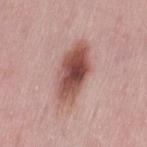Part of a total-body skin-imaging series; this lesion was reviewed on a skin check and was not flagged for biopsy.
A female patient aged approximately 50.
A 15 mm close-up tile from a total-body photography series done for melanoma screening.
This is a white-light tile.
The lesion's longest dimension is about 7 mm.
On the left thigh.